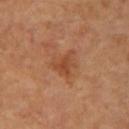<case>
  <biopsy_status>not biopsied; imaged during a skin examination</biopsy_status>
  <image>
    <source>total-body photography crop</source>
    <field_of_view_mm>15</field_of_view_mm>
  </image>
  <automated_metrics>
    <border_irregularity_0_10>4.0</border_irregularity_0_10>
    <color_variation_0_10>4.0</color_variation_0_10>
    <nevus_likeness_0_100>10</nevus_likeness_0_100>
    <lesion_detection_confidence_0_100>100</lesion_detection_confidence_0_100>
  </automated_metrics>
  <site>right thigh</site>
  <lesion_size>
    <long_diameter_mm_approx>3.5</long_diameter_mm_approx>
  </lesion_size>
  <lighting>cross-polarized</lighting>
  <patient>
    <sex>female</sex>
    <age_approx>55</age_approx>
  </patient>
</case>